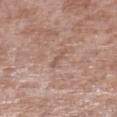A male patient in their mid- to late 50s. A 15 mm close-up tile from a total-body photography series done for melanoma screening. On the left lower leg. The tile uses white-light illumination. The lesion-visualizer software estimated a border-irregularity rating of about 5/10, internal color variation of about 0 on a 0–10 scale, and a peripheral color-asymmetry measure near 0. The analysis additionally found a nevus-likeness score of about 0/100 and a detector confidence of about 90 out of 100 that the crop contains a lesion.